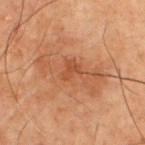• notes: imaged on a skin check; not biopsied
• subject: male, aged around 70
• location: the chest
• illumination: cross-polarized illumination
• acquisition: ~15 mm crop, total-body skin-cancer survey